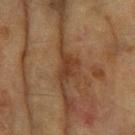Assessment:
No biopsy was performed on this lesion — it was imaged during a full skin examination and was not determined to be concerning.